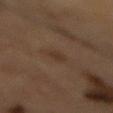– notes — imaged on a skin check; not biopsied
– patient — male, aged around 85 or older
– body site — the back
– image-analysis metrics — a footprint of about 3 mm² and two-axis asymmetry of about 0.2; a mean CIELAB color near L≈28 a*≈13 b*≈23 and a lesion–skin lightness drop of about 5; a classifier nevus-likeness of about 30/100 and lesion-presence confidence of about 100/100
– illumination — cross-polarized
– acquisition — 15 mm crop, total-body photography